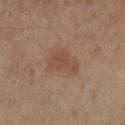follow-up: catalogued during a skin exam; not biopsied
image source: 15 mm crop, total-body photography
anatomic site: the left forearm
subject: female, aged 38–42